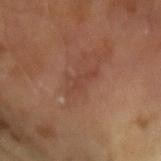{"lesion_size": {"long_diameter_mm_approx": 3.5}, "lighting": "cross-polarized", "patient": {"sex": "male", "age_approx": 65}, "image": {"source": "total-body photography crop", "field_of_view_mm": 15}}